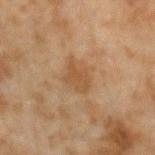Impression: The lesion was photographed on a routine skin check and not biopsied; there is no pathology result. Image and clinical context: The patient is a male aged around 45. The recorded lesion diameter is about 3 mm. An algorithmic analysis of the crop reported roughly 6 lightness units darker than nearby skin. It also reported a border-irregularity rating of about 3/10, internal color variation of about 1.5 on a 0–10 scale, and a peripheral color-asymmetry measure near 0.5. The software also gave a detector confidence of about 100 out of 100 that the crop contains a lesion. The lesion is on the arm. A lesion tile, about 15 mm wide, cut from a 3D total-body photograph. Captured under cross-polarized illumination.